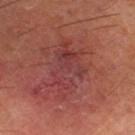Assessment:
Captured during whole-body skin photography for melanoma surveillance; the lesion was not biopsied.
Clinical summary:
The tile uses cross-polarized illumination. The total-body-photography lesion software estimated a lesion color around L≈37 a*≈27 b*≈22 in CIELAB and about 6 CIELAB-L* units darker than the surrounding skin. The analysis additionally found a color-variation rating of about 4.5/10. It also reported a detector confidence of about 100 out of 100 that the crop contains a lesion. A male subject aged 58–62. This image is a 15 mm lesion crop taken from a total-body photograph. On the leg.